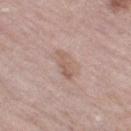No biopsy was performed on this lesion — it was imaged during a full skin examination and was not determined to be concerning. The tile uses white-light illumination. On the right thigh. Approximately 4 mm at its widest. Automated tile analysis of the lesion measured a footprint of about 4.5 mm², an outline eccentricity of about 0.9 (0 = round, 1 = elongated), and a shape-asymmetry score of about 0.35 (0 = symmetric). The analysis additionally found a lesion–skin lightness drop of about 8. The analysis additionally found a lesion-detection confidence of about 100/100. A female patient, roughly 70 years of age. A 15 mm close-up tile from a total-body photography series done for melanoma screening.